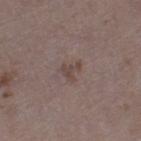biopsy status: no biopsy performed (imaged during a skin exam) | anatomic site: the right thigh | illumination: white-light | image: 15 mm crop, total-body photography | diameter: ~2.5 mm (longest diameter) | image-analysis metrics: a border-irregularity rating of about 6.5/10, a color-variation rating of about 0/10, and peripheral color asymmetry of about 0; an automated nevus-likeness rating near 0 out of 100 and a detector confidence of about 100 out of 100 that the crop contains a lesion | patient: male, roughly 75 years of age.